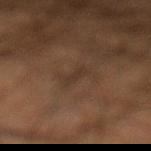workup=no biopsy performed (imaged during a skin exam)
location=the left lower leg
image=15 mm crop, total-body photography
patient=male, aged 53–57